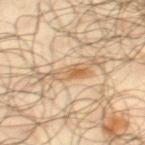{
  "biopsy_status": "not biopsied; imaged during a skin examination",
  "image": {
    "source": "total-body photography crop",
    "field_of_view_mm": 15
  },
  "patient": {
    "sex": "male",
    "age_approx": 65
  },
  "lighting": "cross-polarized",
  "site": "right thigh",
  "lesion_size": {
    "long_diameter_mm_approx": 4.0
  },
  "automated_metrics": {
    "cielab_L": 59,
    "cielab_a": 17,
    "cielab_b": 36,
    "vs_skin_darker_L": 9.0
  }
}